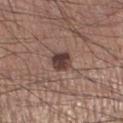| key | value |
|---|---|
| biopsy status | imaged on a skin check; not biopsied |
| lesion diameter | about 2.5 mm |
| image | ~15 mm crop, total-body skin-cancer survey |
| site | the leg |
| patient | male, aged 53–57 |
| tile lighting | white-light |